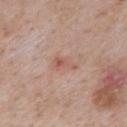| key | value |
|---|---|
| biopsy status | catalogued during a skin exam; not biopsied |
| patient | male, aged around 75 |
| tile lighting | white-light illumination |
| body site | the mid back |
| lesion size | ≈3 mm |
| TBP lesion metrics | a lesion color around L≈56 a*≈23 b*≈27 in CIELAB, about 8 CIELAB-L* units darker than the surrounding skin, and a normalized border contrast of about 5.5; a border-irregularity rating of about 5/10, a within-lesion color-variation index near 2.5/10, and radial color variation of about 0.5; lesion-presence confidence of about 100/100 |
| image source | total-body-photography crop, ~15 mm field of view |